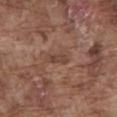Q: Was this lesion biopsied?
A: catalogued during a skin exam; not biopsied
Q: How was the tile lit?
A: white-light illumination
Q: What is the lesion's diameter?
A: ≈2.5 mm
Q: What is the imaging modality?
A: ~15 mm tile from a whole-body skin photo
Q: Where on the body is the lesion?
A: the abdomen
Q: Patient demographics?
A: male, about 75 years old